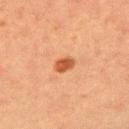No biopsy was performed on this lesion — it was imaged during a full skin examination and was not determined to be concerning. Imaged with cross-polarized lighting. The patient is a female in their mid- to late 50s. Approximately 2.5 mm at its widest. Cropped from a total-body skin-imaging series; the visible field is about 15 mm. From the arm. The lesion-visualizer software estimated a lesion area of about 4 mm². It also reported a nevus-likeness score of about 95/100.